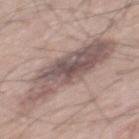The lesion is on the mid back. About 10 mm across. Imaged with white-light lighting. The patient is a male about 55 years old. A 15 mm crop from a total-body photograph taken for skin-cancer surveillance.